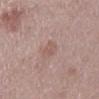workup = imaged on a skin check; not biopsied
image source = 15 mm crop, total-body photography
anatomic site = the mid back
subject = male, aged around 70